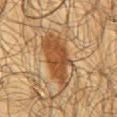Assessment: The lesion was photographed on a routine skin check and not biopsied; there is no pathology result. Context: A male patient aged 63–67. The lesion is on the mid back. The lesion's longest dimension is about 7.5 mm. A lesion tile, about 15 mm wide, cut from a 3D total-body photograph. Imaged with cross-polarized lighting.